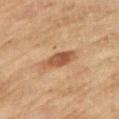Impression: Captured during whole-body skin photography for melanoma surveillance; the lesion was not biopsied. Context: The patient is a female aged 58 to 62. A region of skin cropped from a whole-body photographic capture, roughly 15 mm wide. The lesion is located on the left upper arm.